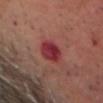The lesion was photographed on a routine skin check and not biopsied; there is no pathology result.